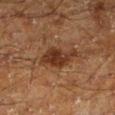follow-up: total-body-photography surveillance lesion; no biopsy | imaging modality: 15 mm crop, total-body photography | illumination: cross-polarized | size: ~5 mm (longest diameter) | image-analysis metrics: an area of roughly 10 mm², an outline eccentricity of about 0.85 (0 = round, 1 = elongated), and two-axis asymmetry of about 0.25; an average lesion color of about L≈26 a*≈17 b*≈24 (CIELAB), a lesion–skin lightness drop of about 9, and a normalized lesion–skin contrast near 9; a border-irregularity rating of about 3/10, internal color variation of about 3.5 on a 0–10 scale, and a peripheral color-asymmetry measure near 1; an automated nevus-likeness rating near 70 out of 100 | patient: male, approximately 60 years of age | body site: the left lower leg.